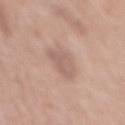Q: Was this lesion biopsied?
A: no biopsy performed (imaged during a skin exam)
Q: What is the imaging modality?
A: ~15 mm tile from a whole-body skin photo
Q: Lesion location?
A: the back
Q: Patient demographics?
A: male, aged 68 to 72